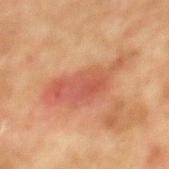Imaged during a routine full-body skin examination; the lesion was not biopsied and no histopathology is available.